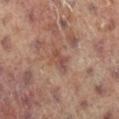The lesion was tiled from a total-body skin photograph and was not biopsied.
A close-up tile cropped from a whole-body skin photograph, about 15 mm across.
The subject is a female about 80 years old.
Imaged with cross-polarized lighting.
The lesion is located on the left leg.
Approximately 3 mm at its widest.